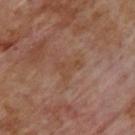Q: Was a biopsy performed?
A: imaged on a skin check; not biopsied
Q: What are the patient's age and sex?
A: male, aged 68 to 72
Q: Lesion location?
A: the upper back
Q: What is the imaging modality?
A: 15 mm crop, total-body photography